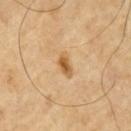The patient is a male about 65 years old. Measured at roughly 3 mm in maximum diameter. Cropped from a total-body skin-imaging series; the visible field is about 15 mm. Automated tile analysis of the lesion measured an average lesion color of about L≈57 a*≈19 b*≈41 (CIELAB) and a normalized border contrast of about 8.5. The analysis additionally found a classifier nevus-likeness of about 80/100 and lesion-presence confidence of about 100/100.